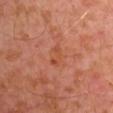Q: Is there a histopathology result?
A: imaged on a skin check; not biopsied
Q: Who is the patient?
A: male, aged 28–32
Q: How large is the lesion?
A: ≈2.5 mm
Q: What lighting was used for the tile?
A: cross-polarized illumination
Q: Lesion location?
A: the left arm
Q: What is the imaging modality?
A: ~15 mm crop, total-body skin-cancer survey